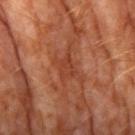No biopsy was performed on this lesion — it was imaged during a full skin examination and was not determined to be concerning.
Approximately 3 mm at its widest.
This is a cross-polarized tile.
A close-up tile cropped from a whole-body skin photograph, about 15 mm across.
A male subject, aged around 60.
Located on the right upper arm.
An algorithmic analysis of the crop reported a shape eccentricity near 0.65 and two-axis asymmetry of about 0.35. The software also gave border irregularity of about 3.5 on a 0–10 scale and a peripheral color-asymmetry measure near 0.5. It also reported an automated nevus-likeness rating near 0 out of 100 and a detector confidence of about 95 out of 100 that the crop contains a lesion.